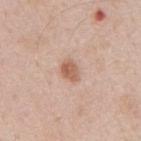The tile uses white-light illumination. A male patient, approximately 50 years of age. The total-body-photography lesion software estimated a border-irregularity index near 2/10, a within-lesion color-variation index near 3/10, and radial color variation of about 1. Longest diameter approximately 3 mm. The lesion is on the back. Cropped from a whole-body photographic skin survey; the tile spans about 15 mm.